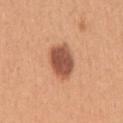The lesion was photographed on a routine skin check and not biopsied; there is no pathology result. Longest diameter approximately 4.5 mm. The lesion is on the arm. A female patient, aged around 30. A 15 mm crop from a total-body photograph taken for skin-cancer surveillance. Imaged with white-light lighting.